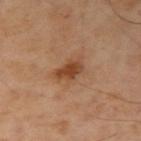Part of a total-body skin-imaging series; this lesion was reviewed on a skin check and was not flagged for biopsy. Cropped from a whole-body photographic skin survey; the tile spans about 15 mm. Measured at roughly 3.5 mm in maximum diameter. A male patient, aged 48 to 52. On the left arm. An algorithmic analysis of the crop reported a border-irregularity index near 3/10, internal color variation of about 2.5 on a 0–10 scale, and radial color variation of about 0.5. It also reported a nevus-likeness score of about 35/100. Imaged with cross-polarized lighting.